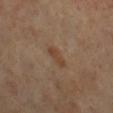Assessment:
Captured during whole-body skin photography for melanoma surveillance; the lesion was not biopsied.
Context:
Captured under cross-polarized illumination. A female subject, in their mid-50s. Measured at roughly 3 mm in maximum diameter. A close-up tile cropped from a whole-body skin photograph, about 15 mm across. Automated tile analysis of the lesion measured a lesion area of about 3 mm², a shape eccentricity near 0.95, and a symmetry-axis asymmetry near 0.3. The analysis additionally found a lesion color around L≈43 a*≈18 b*≈30 in CIELAB and a lesion-to-skin contrast of about 7 (normalized; higher = more distinct). And it measured a lesion-detection confidence of about 100/100. The lesion is on the right lower leg.